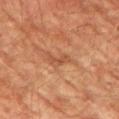Recorded during total-body skin imaging; not selected for excision or biopsy. The lesion-visualizer software estimated border irregularity of about 6 on a 0–10 scale, a within-lesion color-variation index near 0/10, and a peripheral color-asymmetry measure near 0. It also reported a nevus-likeness score of about 0/100. About 2.5 mm across. Captured under cross-polarized illumination. On the left upper arm. A male patient about 75 years old. A 15 mm crop from a total-body photograph taken for skin-cancer surveillance.